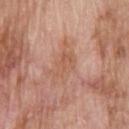Part of a total-body skin-imaging series; this lesion was reviewed on a skin check and was not flagged for biopsy.